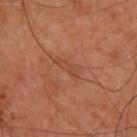follow-up = imaged on a skin check; not biopsied
acquisition = total-body-photography crop, ~15 mm field of view
subject = male, aged 58–62
body site = the back
diameter = about 3.5 mm
tile lighting = cross-polarized illumination